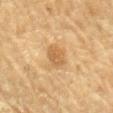Q: Was this lesion biopsied?
A: imaged on a skin check; not biopsied
Q: What is the anatomic site?
A: the mid back
Q: What did automated image analysis measure?
A: a shape eccentricity near 0.55; an average lesion color of about L≈53 a*≈17 b*≈37 (CIELAB), a lesion–skin lightness drop of about 8, and a normalized border contrast of about 6.5; a detector confidence of about 100 out of 100 that the crop contains a lesion
Q: What is the lesion's diameter?
A: about 3 mm
Q: How was the tile lit?
A: cross-polarized illumination
Q: What is the imaging modality?
A: ~15 mm crop, total-body skin-cancer survey
Q: What are the patient's age and sex?
A: male, aged 83 or over